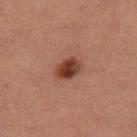biopsy_status: not biopsied; imaged during a skin examination
lighting: cross-polarized
automated_metrics:
  area_mm2_approx: 6.0
  eccentricity: 0.7
  shape_asymmetry: 0.2
  cielab_L: 31
  cielab_a: 21
  cielab_b: 23
  vs_skin_darker_L: 11.0
  vs_skin_contrast_norm: 10.5
site: leg
patient:
  sex: female
  age_approx: 55
image:
  source: total-body photography crop
  field_of_view_mm: 15